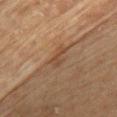| key | value |
|---|---|
| notes | total-body-photography surveillance lesion; no biopsy |
| TBP lesion metrics | a mean CIELAB color near L≈41 a*≈17 b*≈29, roughly 7 lightness units darker than nearby skin, and a lesion-to-skin contrast of about 5.5 (normalized; higher = more distinct); a border-irregularity rating of about 5.5/10 and radial color variation of about 0; an automated nevus-likeness rating near 0 out of 100 and a detector confidence of about 65 out of 100 that the crop contains a lesion |
| body site | the chest |
| patient | female, aged 78–82 |
| image source | total-body-photography crop, ~15 mm field of view |
| illumination | cross-polarized |
| size | ~2.5 mm (longest diameter) |